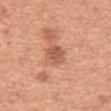Findings:
• lesion diameter: ~3.5 mm (longest diameter)
• anatomic site: the abdomen
• automated metrics: a normalized border contrast of about 7; a color-variation rating of about 4/10
• acquisition: 15 mm crop, total-body photography
• subject: male, approximately 65 years of age
• illumination: white-light illumination